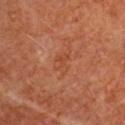Clinical impression: The lesion was tiled from a total-body skin photograph and was not biopsied. Image and clinical context: The subject is a male approximately 65 years of age. The lesion is located on the right upper arm. A roughly 15 mm field-of-view crop from a total-body skin photograph.